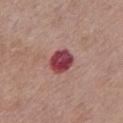Impression:
No biopsy was performed on this lesion — it was imaged during a full skin examination and was not determined to be concerning.
Background:
Imaged with white-light lighting. Cropped from a whole-body photographic skin survey; the tile spans about 15 mm. A female subject in their mid- to late 60s. From the chest.